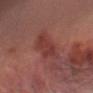Clinical impression: The lesion was photographed on a routine skin check and not biopsied; there is no pathology result. Context: The lesion is located on the right arm. This is a cross-polarized tile. Longest diameter approximately 5.5 mm. A close-up tile cropped from a whole-body skin photograph, about 15 mm across. A male patient, aged approximately 60.